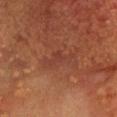{
  "biopsy_status": "not biopsied; imaged during a skin examination",
  "lesion_size": {
    "long_diameter_mm_approx": 4.5
  },
  "lighting": "cross-polarized",
  "image": {
    "source": "total-body photography crop",
    "field_of_view_mm": 15
  },
  "patient": {
    "sex": "female",
    "age_approx": 40
  },
  "site": "chest"
}